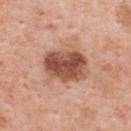Clinical summary:
Located on the back. The tile uses white-light illumination. The patient is a female about 50 years old. About 5 mm across. A 15 mm close-up tile from a total-body photography series done for melanoma screening.